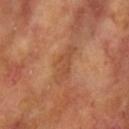Notes:
– workup: no biopsy performed (imaged during a skin exam)
– lighting: cross-polarized illumination
– patient: female, in their mid- to late 70s
– image source: ~15 mm tile from a whole-body skin photo
– location: the arm